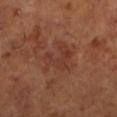Q: Was this lesion biopsied?
A: no biopsy performed (imaged during a skin exam)
Q: What are the patient's age and sex?
A: male, aged approximately 65
Q: What kind of image is this?
A: 15 mm crop, total-body photography
Q: How large is the lesion?
A: ~4 mm (longest diameter)
Q: Where on the body is the lesion?
A: the left lower leg
Q: Illumination type?
A: cross-polarized